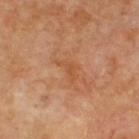workup — catalogued during a skin exam; not biopsied | location — the upper back | diameter — about 3 mm | tile lighting — cross-polarized illumination | image — 15 mm crop, total-body photography | image-analysis metrics — a border-irregularity index near 6.5/10, a color-variation rating of about 0.5/10, and peripheral color asymmetry of about 0 | subject — male, aged approximately 70.